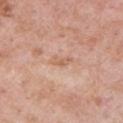This lesion was catalogued during total-body skin photography and was not selected for biopsy. The tile uses white-light illumination. Cropped from a total-body skin-imaging series; the visible field is about 15 mm. The patient is a male about 55 years old. The lesion's longest dimension is about 3 mm. On the left upper arm.